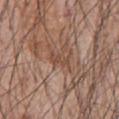This lesion was catalogued during total-body skin photography and was not selected for biopsy.
A male subject roughly 55 years of age.
Captured under white-light illumination.
Cropped from a total-body skin-imaging series; the visible field is about 15 mm.
On the chest.
The lesion-visualizer software estimated a shape eccentricity near 0.85. It also reported a lesion color around L≈47 a*≈20 b*≈28 in CIELAB and roughly 7 lightness units darker than nearby skin.